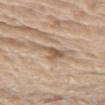biopsy_status: not biopsied; imaged during a skin examination
lighting: white-light
image:
  source: total-body photography crop
  field_of_view_mm: 15
patient:
  sex: male
  age_approx: 70
site: left thigh